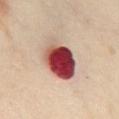  biopsy_status: not biopsied; imaged during a skin examination
  patient:
    sex: female
    age_approx: 55
  image:
    source: total-body photography crop
    field_of_view_mm: 15
  site: mid back